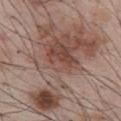biopsy_status: not biopsied; imaged during a skin examination
image:
  source: total-body photography crop
  field_of_view_mm: 15
patient:
  sex: male
  age_approx: 55
site: abdomen